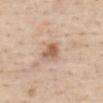Q: Was this lesion biopsied?
A: total-body-photography surveillance lesion; no biopsy
Q: Automated lesion metrics?
A: a shape eccentricity near 0.65 and two-axis asymmetry of about 0.3; a mean CIELAB color near L≈59 a*≈20 b*≈30, about 12 CIELAB-L* units darker than the surrounding skin, and a lesion-to-skin contrast of about 8 (normalized; higher = more distinct); a border-irregularity index near 2.5/10
Q: Illumination type?
A: white-light
Q: Lesion size?
A: ~2.5 mm (longest diameter)
Q: What are the patient's age and sex?
A: female, aged approximately 65
Q: What is the imaging modality?
A: 15 mm crop, total-body photography
Q: What is the anatomic site?
A: the abdomen